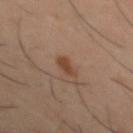Imaged during a routine full-body skin examination; the lesion was not biopsied and no histopathology is available.
The lesion is on the mid back.
The subject is a male in their 40s.
A close-up tile cropped from a whole-body skin photograph, about 15 mm across.
The recorded lesion diameter is about 2.5 mm.
The lesion-visualizer software estimated a mean CIELAB color near L≈42 a*≈20 b*≈29 and a normalized lesion–skin contrast near 8.
The tile uses cross-polarized illumination.